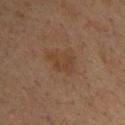Captured during whole-body skin photography for melanoma surveillance; the lesion was not biopsied.
A male patient, roughly 35 years of age.
Located on the back.
A roughly 15 mm field-of-view crop from a total-body skin photograph.